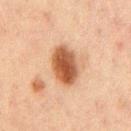Impression:
The lesion was photographed on a routine skin check and not biopsied; there is no pathology result.
Background:
The lesion is located on the chest. A male subject in their 50s. Captured under cross-polarized illumination. A lesion tile, about 15 mm wide, cut from a 3D total-body photograph. About 5 mm across. The lesion-visualizer software estimated a lesion area of about 12 mm², an outline eccentricity of about 0.75 (0 = round, 1 = elongated), and a shape-asymmetry score of about 0.1 (0 = symmetric). And it measured an average lesion color of about L≈43 a*≈20 b*≈30 (CIELAB) and a normalized lesion–skin contrast near 11. The software also gave a border-irregularity rating of about 1.5/10, a within-lesion color-variation index near 5/10, and a peripheral color-asymmetry measure near 1.5. The software also gave an automated nevus-likeness rating near 100 out of 100.